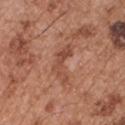No biopsy was performed on this lesion — it was imaged during a full skin examination and was not determined to be concerning.
The subject is a male in their mid-50s.
A close-up tile cropped from a whole-body skin photograph, about 15 mm across.
Measured at roughly 4.5 mm in maximum diameter.
On the chest.